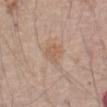Captured during whole-body skin photography for melanoma surveillance; the lesion was not biopsied.
A 15 mm close-up tile from a total-body photography series done for melanoma screening.
The subject is a male aged around 80.
Captured under white-light illumination.
From the abdomen.
Measured at roughly 2.5 mm in maximum diameter.